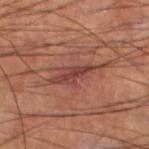No biopsy was performed on this lesion — it was imaged during a full skin examination and was not determined to be concerning. Cropped from a total-body skin-imaging series; the visible field is about 15 mm. This is a cross-polarized tile. From the left lower leg. Automated tile analysis of the lesion measured a footprint of about 8 mm², an outline eccentricity of about 0.95 (0 = round, 1 = elongated), and a shape-asymmetry score of about 0.35 (0 = symmetric). The analysis additionally found a detector confidence of about 60 out of 100 that the crop contains a lesion. A male subject approximately 60 years of age. The recorded lesion diameter is about 6 mm.